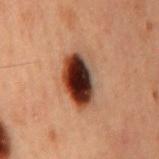Q: Is there a histopathology result?
A: total-body-photography surveillance lesion; no biopsy
Q: What are the patient's age and sex?
A: male, roughly 60 years of age
Q: What is the anatomic site?
A: the mid back
Q: What kind of image is this?
A: ~15 mm crop, total-body skin-cancer survey